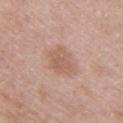Imaged during a routine full-body skin examination; the lesion was not biopsied and no histopathology is available. A 15 mm close-up tile from a total-body photography series done for melanoma screening. The lesion is located on the right upper arm. A female patient, aged approximately 30. This is a white-light tile. Automated image analysis of the tile measured an outline eccentricity of about 0.5 (0 = round, 1 = elongated) and a symmetry-axis asymmetry near 0.2. It also reported lesion-presence confidence of about 100/100.